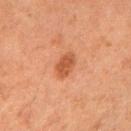{
  "biopsy_status": "not biopsied; imaged during a skin examination",
  "patient": {
    "sex": "female",
    "age_approx": 60
  },
  "automated_metrics": {
    "eccentricity": 0.6,
    "shape_asymmetry": 0.2,
    "cielab_L": 44,
    "cielab_a": 24,
    "cielab_b": 33,
    "vs_skin_darker_L": 9.0,
    "vs_skin_contrast_norm": 7.5
  },
  "lesion_size": {
    "long_diameter_mm_approx": 3.0
  },
  "image": {
    "source": "total-body photography crop",
    "field_of_view_mm": 15
  },
  "site": "right forearm",
  "lighting": "cross-polarized"
}